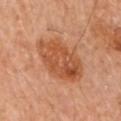workup: catalogued during a skin exam; not biopsied | illumination: cross-polarized illumination | subject: female, in their 60s | location: the left arm | diameter: about 6 mm | acquisition: ~15 mm tile from a whole-body skin photo.